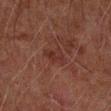Case summary:
* follow-up — total-body-photography surveillance lesion; no biopsy